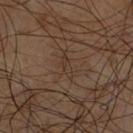Located on the chest. The total-body-photography lesion software estimated a footprint of about 2.5 mm², an outline eccentricity of about 0.95 (0 = round, 1 = elongated), and a symmetry-axis asymmetry near 0.35. And it measured a mean CIELAB color near L≈30 a*≈13 b*≈22 and about 4 CIELAB-L* units darker than the surrounding skin. This is a cross-polarized tile. A male patient, in their 60s. A 15 mm close-up extracted from a 3D total-body photography capture. The lesion's longest dimension is about 3 mm.